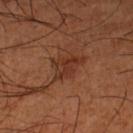Captured during whole-body skin photography for melanoma surveillance; the lesion was not biopsied.
The subject is a male aged approximately 65.
The tile uses cross-polarized illumination.
Longest diameter approximately 4 mm.
The lesion is located on the left lower leg.
A close-up tile cropped from a whole-body skin photograph, about 15 mm across.